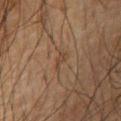Q: Illumination type?
A: cross-polarized
Q: Who is the patient?
A: male, roughly 65 years of age
Q: What is the imaging modality?
A: total-body-photography crop, ~15 mm field of view
Q: Automated lesion metrics?
A: an outline eccentricity of about 0.9 (0 = round, 1 = elongated) and two-axis asymmetry of about 0.4; a lesion color around L≈34 a*≈14 b*≈24 in CIELAB and a normalized lesion–skin contrast near 5; a nevus-likeness score of about 0/100 and a detector confidence of about 50 out of 100 that the crop contains a lesion
Q: What is the anatomic site?
A: the chest